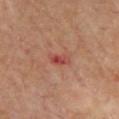Notes:
• notes — catalogued during a skin exam; not biopsied
• diameter — about 2.5 mm
• patient — male, aged 68–72
• site — the front of the torso
• acquisition — ~15 mm tile from a whole-body skin photo